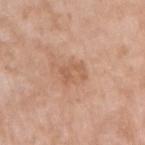<record>
<biopsy_status>not biopsied; imaged during a skin examination</biopsy_status>
<automated_metrics>
  <area_mm2_approx>5.5</area_mm2_approx>
  <eccentricity>0.65</eccentricity>
  <shape_asymmetry>0.3</shape_asymmetry>
  <cielab_L>59</cielab_L>
  <cielab_a>21</cielab_a>
  <cielab_b>32</cielab_b>
  <vs_skin_darker_L>7.0</vs_skin_darker_L>
  <vs_skin_contrast_norm>5.0</vs_skin_contrast_norm>
  <border_irregularity_0_10>5.5</border_irregularity_0_10>
  <color_variation_0_10>1.0</color_variation_0_10>
  <peripheral_color_asymmetry>0.5</peripheral_color_asymmetry>
  <lesion_detection_confidence_0_100>100</lesion_detection_confidence_0_100>
</automated_metrics>
<lesion_size>
  <long_diameter_mm_approx>3.0</long_diameter_mm_approx>
</lesion_size>
<patient>
  <sex>female</sex>
  <age_approx>75</age_approx>
</patient>
<image>
  <source>total-body photography crop</source>
  <field_of_view_mm>15</field_of_view_mm>
</image>
<lighting>white-light</lighting>
<site>right upper arm</site>
</record>